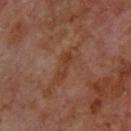{
  "biopsy_status": "not biopsied; imaged during a skin examination",
  "patient": {
    "sex": "male",
    "age_approx": 70
  },
  "lesion_size": {
    "long_diameter_mm_approx": 5.0
  },
  "image": {
    "source": "total-body photography crop",
    "field_of_view_mm": 15
  },
  "site": "upper back",
  "automated_metrics": {
    "color_variation_0_10": 2.0
  },
  "lighting": "cross-polarized"
}